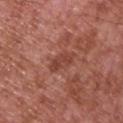Q: Was this lesion biopsied?
A: imaged on a skin check; not biopsied
Q: Patient demographics?
A: male, aged around 65
Q: Lesion location?
A: the chest
Q: What kind of image is this?
A: 15 mm crop, total-body photography